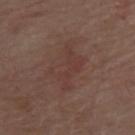Captured during whole-body skin photography for melanoma surveillance; the lesion was not biopsied.
Approximately 5.5 mm at its widest.
A lesion tile, about 15 mm wide, cut from a 3D total-body photograph.
The subject is a male in their 70s.
Located on the upper back.
This is a white-light tile.